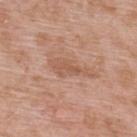Part of a total-body skin-imaging series; this lesion was reviewed on a skin check and was not flagged for biopsy. A region of skin cropped from a whole-body photographic capture, roughly 15 mm wide. A male subject, in their 50s. The lesion is located on the back. The recorded lesion diameter is about 5.5 mm. Captured under white-light illumination.